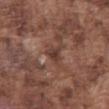biopsy status: total-body-photography surveillance lesion; no biopsy | location: the abdomen | tile lighting: white-light | subject: male, in their mid-70s | imaging modality: 15 mm crop, total-body photography | size: ≈2.5 mm.